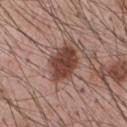<record>
<biopsy_status>not biopsied; imaged during a skin examination</biopsy_status>
<patient>
  <sex>male</sex>
  <age_approx>55</age_approx>
</patient>
<image>
  <source>total-body photography crop</source>
  <field_of_view_mm>15</field_of_view_mm>
</image>
<lesion_size>
  <long_diameter_mm_approx>5.0</long_diameter_mm_approx>
</lesion_size>
<lighting>white-light</lighting>
<site>chest</site>
<automated_metrics>
  <area_mm2_approx>12.0</area_mm2_approx>
  <shape_asymmetry>0.2</shape_asymmetry>
  <vs_skin_darker_L>14.0</vs_skin_darker_L>
  <vs_skin_contrast_norm>10.5</vs_skin_contrast_norm>
  <border_irregularity_0_10>2.0</border_irregularity_0_10>
  <color_variation_0_10>4.0</color_variation_0_10>
  <peripheral_color_asymmetry>1.0</peripheral_color_asymmetry>
  <lesion_detection_confidence_0_100>100</lesion_detection_confidence_0_100>
</automated_metrics>
</record>